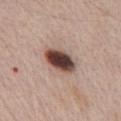workup: no biopsy performed (imaged during a skin exam)
TBP lesion metrics: a border-irregularity index near 1.5/10
location: the chest
lighting: white-light illumination
patient: male, about 65 years old
image source: ~15 mm crop, total-body skin-cancer survey